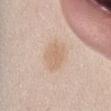The total-body-photography lesion software estimated a lesion area of about 8.5 mm², an eccentricity of roughly 0.7, and a shape-asymmetry score of about 0.15 (0 = symmetric). And it measured a nevus-likeness score of about 10/100.
Imaged with white-light lighting.
A region of skin cropped from a whole-body photographic capture, roughly 15 mm wide.
The subject is a female aged around 25.
On the abdomen.
The lesion's longest dimension is about 4 mm.